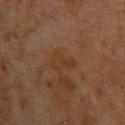{
  "biopsy_status": "not biopsied; imaged during a skin examination",
  "automated_metrics": {
    "area_mm2_approx": 5.0,
    "eccentricity": 0.85,
    "shape_asymmetry": 0.45,
    "cielab_L": 26,
    "cielab_a": 15,
    "cielab_b": 25,
    "nevus_likeness_0_100": 0
  },
  "lighting": "cross-polarized",
  "lesion_size": {
    "long_diameter_mm_approx": 3.5
  },
  "site": "upper back",
  "image": {
    "source": "total-body photography crop",
    "field_of_view_mm": 15
  },
  "patient": {
    "sex": "male",
    "age_approx": 60
  }
}